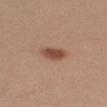Q: Was this lesion biopsied?
A: imaged on a skin check; not biopsied
Q: What is the imaging modality?
A: 15 mm crop, total-body photography
Q: How large is the lesion?
A: ~3 mm (longest diameter)
Q: What are the patient's age and sex?
A: male, aged 33 to 37
Q: Illumination type?
A: white-light illumination
Q: Lesion location?
A: the arm